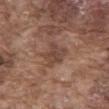Clinical impression: The lesion was tiled from a total-body skin photograph and was not biopsied. Clinical summary: This image is a 15 mm lesion crop taken from a total-body photograph. The recorded lesion diameter is about 3 mm. Automated tile analysis of the lesion measured a mean CIELAB color near L≈44 a*≈18 b*≈25 and a normalized lesion–skin contrast near 6.5. The software also gave a border-irregularity index near 4/10, a color-variation rating of about 3/10, and a peripheral color-asymmetry measure near 1. And it measured an automated nevus-likeness rating near 0 out of 100 and a lesion-detection confidence of about 85/100. The lesion is on the abdomen. A male subject, in their mid-70s. Imaged with white-light lighting.